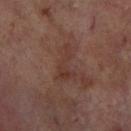A male patient roughly 70 years of age.
Located on the right lower leg.
Longest diameter approximately 4.5 mm.
This image is a 15 mm lesion crop taken from a total-body photograph.
This is a cross-polarized tile.